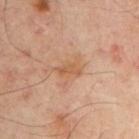Part of a total-body skin-imaging series; this lesion was reviewed on a skin check and was not flagged for biopsy.
The patient is a male aged 48 to 52.
Measured at roughly 2.5 mm in maximum diameter.
Cropped from a total-body skin-imaging series; the visible field is about 15 mm.
The lesion is located on the front of the torso.
Automated tile analysis of the lesion measured an area of roughly 4 mm², a shape eccentricity near 0.8, and a shape-asymmetry score of about 0.35 (0 = symmetric). It also reported a border-irregularity index near 3.5/10.